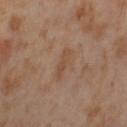biopsy status: catalogued during a skin exam; not biopsied
subject: female, about 55 years old
body site: the left thigh
lesion diameter: ≈3.5 mm
image source: ~15 mm tile from a whole-body skin photo
illumination: cross-polarized illumination